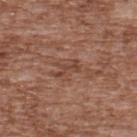Q: Was this lesion biopsied?
A: no biopsy performed (imaged during a skin exam)
Q: What is the imaging modality?
A: ~15 mm tile from a whole-body skin photo
Q: Where on the body is the lesion?
A: the upper back
Q: How large is the lesion?
A: ≈3 mm
Q: What lighting was used for the tile?
A: white-light
Q: Patient demographics?
A: male, about 75 years old